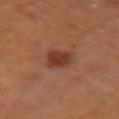Recorded during total-body skin imaging; not selected for excision or biopsy.
Longest diameter approximately 4 mm.
The patient is a male in their mid- to late 60s.
A lesion tile, about 15 mm wide, cut from a 3D total-body photograph.
Located on the arm.
Captured under cross-polarized illumination.
Automated tile analysis of the lesion measured border irregularity of about 2 on a 0–10 scale, internal color variation of about 2.5 on a 0–10 scale, and radial color variation of about 1.